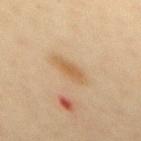{"biopsy_status": "not biopsied; imaged during a skin examination", "image": {"source": "total-body photography crop", "field_of_view_mm": 15}, "automated_metrics": {"shape_asymmetry": 0.2, "cielab_L": 52, "cielab_a": 15, "cielab_b": 33, "vs_skin_darker_L": 7.0, "vs_skin_contrast_norm": 6.5, "nevus_likeness_0_100": 65, "lesion_detection_confidence_0_100": 100}, "patient": {"sex": "female", "age_approx": 45}, "lesion_size": {"long_diameter_mm_approx": 4.0}, "lighting": "cross-polarized", "site": "back"}